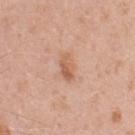Context: The subject is a male aged around 55. A roughly 15 mm field-of-view crop from a total-body skin photograph. The lesion is located on the chest.